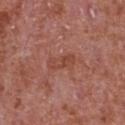{"biopsy_status": "not biopsied; imaged during a skin examination", "site": "chest", "automated_metrics": {"area_mm2_approx": 5.5, "shape_asymmetry": 0.25, "cielab_L": 46, "cielab_a": 26, "cielab_b": 28, "vs_skin_darker_L": 6.0, "nevus_likeness_0_100": 0, "lesion_detection_confidence_0_100": 100}, "lighting": "white-light", "lesion_size": {"long_diameter_mm_approx": 3.5}, "image": {"source": "total-body photography crop", "field_of_view_mm": 15}, "patient": {"sex": "male", "age_approx": 65}}